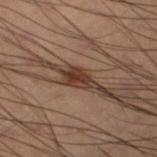Q: Illumination type?
A: cross-polarized
Q: How large is the lesion?
A: ≈3 mm
Q: Where on the body is the lesion?
A: the left thigh
Q: How was this image acquired?
A: 15 mm crop, total-body photography
Q: Who is the patient?
A: male, in their mid-50s